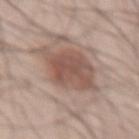The lesion was photographed on a routine skin check and not biopsied; there is no pathology result.
The patient is a male aged 53 to 57.
A 15 mm crop from a total-body photograph taken for skin-cancer surveillance.
Longest diameter approximately 5.5 mm.
On the front of the torso.